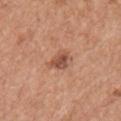Recorded during total-body skin imaging; not selected for excision or biopsy.
From the arm.
A male subject, aged 63 to 67.
Cropped from a whole-body photographic skin survey; the tile spans about 15 mm.
The tile uses white-light illumination.